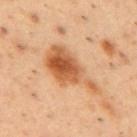Case summary:
• workup · imaged on a skin check; not biopsied
• site · the upper back
• patient · male, aged 53–57
• tile lighting · cross-polarized
• acquisition · ~15 mm tile from a whole-body skin photo